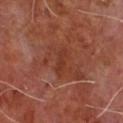subject: male, in their mid-60s
image: 15 mm crop, total-body photography
body site: the chest